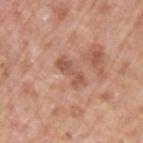No biopsy was performed on this lesion — it was imaged during a full skin examination and was not determined to be concerning.
The subject is a male roughly 70 years of age.
This is a white-light tile.
Longest diameter approximately 3.5 mm.
The lesion-visualizer software estimated border irregularity of about 5 on a 0–10 scale, a within-lesion color-variation index near 2.5/10, and peripheral color asymmetry of about 0.5.
A close-up tile cropped from a whole-body skin photograph, about 15 mm across.
The lesion is on the left upper arm.